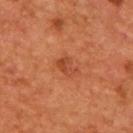The lesion-visualizer software estimated a footprint of about 3.5 mm² and an eccentricity of roughly 0.75. The software also gave a color-variation rating of about 2/10 and peripheral color asymmetry of about 0.5. And it measured a nevus-likeness score of about 15/100 and a lesion-detection confidence of about 100/100. The lesion is located on the chest. Cropped from a total-body skin-imaging series; the visible field is about 15 mm. The patient is a male in their mid-50s. The tile uses cross-polarized illumination. The lesion's longest dimension is about 2.5 mm.